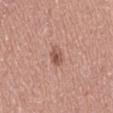<case>
  <biopsy_status>not biopsied; imaged during a skin examination</biopsy_status>
  <lighting>white-light</lighting>
  <patient>
    <sex>female</sex>
    <age_approx>50</age_approx>
  </patient>
  <automated_metrics>
    <eccentricity>0.85</eccentricity>
    <shape_asymmetry>0.2</shape_asymmetry>
    <vs_skin_darker_L>10.0</vs_skin_darker_L>
    <vs_skin_contrast_norm>7.0</vs_skin_contrast_norm>
    <lesion_detection_confidence_0_100>100</lesion_detection_confidence_0_100>
  </automated_metrics>
  <image>
    <source>total-body photography crop</source>
    <field_of_view_mm>15</field_of_view_mm>
  </image>
  <lesion_size>
    <long_diameter_mm_approx>3.0</long_diameter_mm_approx>
  </lesion_size>
  <site>leg</site>
</case>